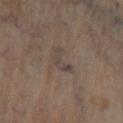The lesion was tiled from a total-body skin photograph and was not biopsied.